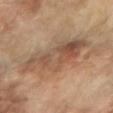Assessment:
No biopsy was performed on this lesion — it was imaged during a full skin examination and was not determined to be concerning.
Context:
On the right forearm. A 15 mm crop from a total-body photograph taken for skin-cancer surveillance. A female patient aged 68–72.